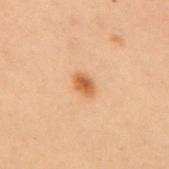workup: imaged on a skin check; not biopsied | patient: female, about 50 years old | site: the upper back | automated metrics: a footprint of about 3.5 mm²; an automated nevus-likeness rating near 95 out of 100 and a lesion-detection confidence of about 100/100 | lesion size: about 2.5 mm | image: ~15 mm tile from a whole-body skin photo | lighting: cross-polarized illumination.